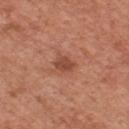This lesion was catalogued during total-body skin photography and was not selected for biopsy. The recorded lesion diameter is about 2.5 mm. The subject is a male aged 63 to 67. Cropped from a whole-body photographic skin survey; the tile spans about 15 mm. On the chest. Automated tile analysis of the lesion measured a footprint of about 4 mm² and two-axis asymmetry of about 0.25. The analysis additionally found a border-irregularity index near 2.5/10, a color-variation rating of about 1.5/10, and peripheral color asymmetry of about 0.5. And it measured lesion-presence confidence of about 100/100.